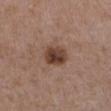Findings:
• follow-up — no biopsy performed (imaged during a skin exam)